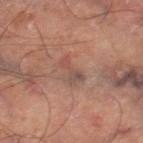Imaged during a routine full-body skin examination; the lesion was not biopsied and no histopathology is available.
An algorithmic analysis of the crop reported an area of roughly 3.5 mm² and an eccentricity of roughly 0.9. It also reported a classifier nevus-likeness of about 0/100 and a detector confidence of about 100 out of 100 that the crop contains a lesion.
A 15 mm close-up extracted from a 3D total-body photography capture.
Located on the right thigh.
Captured under cross-polarized illumination.
Measured at roughly 3 mm in maximum diameter.